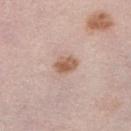Impression:
The lesion was photographed on a routine skin check and not biopsied; there is no pathology result.
Clinical summary:
The tile uses white-light illumination. A female patient aged 63–67. Located on the right lower leg. About 3 mm across. A 15 mm crop from a total-body photograph taken for skin-cancer surveillance.